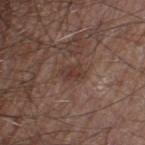Findings:
- workup: imaged on a skin check; not biopsied
- lesion size: ~3 mm (longest diameter)
- patient: male, in their 60s
- tile lighting: white-light illumination
- location: the left thigh
- TBP lesion metrics: a lesion area of about 4 mm² and a shape-asymmetry score of about 0.35 (0 = symmetric); a lesion–skin lightness drop of about 7
- imaging modality: ~15 mm tile from a whole-body skin photo